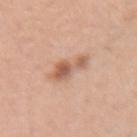  biopsy_status: not biopsied; imaged during a skin examination
  image:
    source: total-body photography crop
    field_of_view_mm: 15
  site: right forearm
  lighting: white-light
  patient:
    sex: female
    age_approx: 40